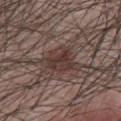Q: Was this lesion biopsied?
A: imaged on a skin check; not biopsied
Q: Automated lesion metrics?
A: a lesion area of about 9 mm², a shape eccentricity near 0.35, and two-axis asymmetry of about 0.25; a lesion–skin lightness drop of about 8 and a normalized lesion–skin contrast near 8; a border-irregularity rating of about 2.5/10, internal color variation of about 4.5 on a 0–10 scale, and a peripheral color-asymmetry measure near 1.5; an automated nevus-likeness rating near 60 out of 100 and a lesion-detection confidence of about 100/100
Q: How was this image acquired?
A: 15 mm crop, total-body photography
Q: How was the tile lit?
A: white-light
Q: What is the anatomic site?
A: the chest
Q: What are the patient's age and sex?
A: male, about 40 years old
Q: How large is the lesion?
A: ~3.5 mm (longest diameter)